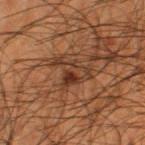follow-up: catalogued during a skin exam; not biopsied | image-analysis metrics: a lesion color around L≈29 a*≈18 b*≈25 in CIELAB, a lesion–skin lightness drop of about 8, and a normalized border contrast of about 8; border irregularity of about 9 on a 0–10 scale, a color-variation rating of about 9/10, and peripheral color asymmetry of about 3; a nevus-likeness score of about 45/100 and a lesion-detection confidence of about 75/100 | illumination: cross-polarized | site: the left thigh | size: ≈4.5 mm | patient: male, in their mid-60s | image source: ~15 mm tile from a whole-body skin photo.